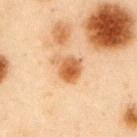{
  "biopsy_status": "not biopsied; imaged during a skin examination",
  "image": {
    "source": "total-body photography crop",
    "field_of_view_mm": 15
  },
  "site": "left upper arm",
  "lighting": "cross-polarized",
  "patient": {
    "sex": "male",
    "age_approx": 55
  },
  "automated_metrics": {
    "shape_asymmetry": 0.3,
    "cielab_L": 51,
    "cielab_a": 21,
    "cielab_b": 35,
    "vs_skin_darker_L": 12.0,
    "vs_skin_contrast_norm": 9.0,
    "border_irregularity_0_10": 2.5,
    "peripheral_color_asymmetry": 2.0,
    "nevus_likeness_0_100": 95
  }
}